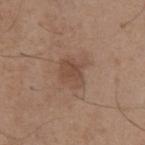Impression: Captured during whole-body skin photography for melanoma surveillance; the lesion was not biopsied. Background: The lesion's longest dimension is about 3.5 mm. A male patient roughly 65 years of age. The tile uses white-light illumination. An algorithmic analysis of the crop reported a footprint of about 8.5 mm², an eccentricity of roughly 0.4, and two-axis asymmetry of about 0.25. The software also gave roughly 7 lightness units darker than nearby skin and a normalized border contrast of about 5.5. The analysis additionally found a border-irregularity index near 3/10, a within-lesion color-variation index near 2.5/10, and radial color variation of about 1. It also reported an automated nevus-likeness rating near 0 out of 100 and lesion-presence confidence of about 100/100. A region of skin cropped from a whole-body photographic capture, roughly 15 mm wide. The lesion is located on the abdomen.